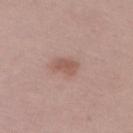notes: catalogued during a skin exam; not biopsied | subject: female, about 50 years old | acquisition: ~15 mm tile from a whole-body skin photo | body site: the right upper arm | illumination: white-light illumination | image-analysis metrics: border irregularity of about 2.5 on a 0–10 scale, a color-variation rating of about 2/10, and a peripheral color-asymmetry measure near 0.5.